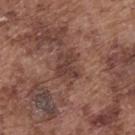biopsy_status: not biopsied; imaged during a skin examination
patient:
  sex: male
  age_approx: 75
automated_metrics:
  area_mm2_approx: 9.0
  eccentricity: 0.7
  shape_asymmetry: 0.3
  nevus_likeness_0_100: 10
lighting: white-light
lesion_size:
  long_diameter_mm_approx: 4.0
site: upper back
image:
  source: total-body photography crop
  field_of_view_mm: 15